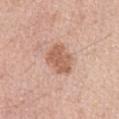Findings:
* automated lesion analysis · a border-irregularity index near 3/10, internal color variation of about 2 on a 0–10 scale, and a peripheral color-asymmetry measure near 0.5; a nevus-likeness score of about 20/100 and a detector confidence of about 100 out of 100 that the crop contains a lesion
* body site · the abdomen
* size · ~4 mm (longest diameter)
* imaging modality · 15 mm crop, total-body photography
* subject · male, about 70 years old